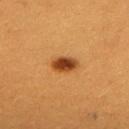Assessment: Imaged during a routine full-body skin examination; the lesion was not biopsied and no histopathology is available. Background: The patient is a female roughly 30 years of age. A roughly 15 mm field-of-view crop from a total-body skin photograph. Longest diameter approximately 3 mm. Captured under cross-polarized illumination. From the upper back.